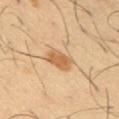The lesion was tiled from a total-body skin photograph and was not biopsied. Measured at roughly 3 mm in maximum diameter. A region of skin cropped from a whole-body photographic capture, roughly 15 mm wide. Automated image analysis of the tile measured a lesion area of about 6 mm², a shape eccentricity near 0.6, and a shape-asymmetry score of about 0.2 (0 = symmetric). It also reported a lesion color around L≈60 a*≈20 b*≈38 in CIELAB, about 11 CIELAB-L* units darker than the surrounding skin, and a lesion-to-skin contrast of about 7.5 (normalized; higher = more distinct). It also reported a border-irregularity index near 2/10 and peripheral color asymmetry of about 1. The patient is a male aged 38 to 42. The tile uses cross-polarized illumination. The lesion is on the left upper arm.